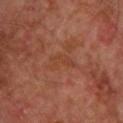<case>
<biopsy_status>not biopsied; imaged during a skin examination</biopsy_status>
<image>
  <source>total-body photography crop</source>
  <field_of_view_mm>15</field_of_view_mm>
</image>
<lesion_size>
  <long_diameter_mm_approx>3.5</long_diameter_mm_approx>
</lesion_size>
<lighting>cross-polarized</lighting>
<patient>
  <sex>male</sex>
  <age_approx>70</age_approx>
</patient>
<site>back</site>
</case>